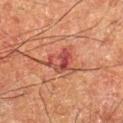This lesion was catalogued during total-body skin photography and was not selected for biopsy. The tile uses cross-polarized illumination. Approximately 4 mm at its widest. A close-up tile cropped from a whole-body skin photograph, about 15 mm across. A male patient, aged around 60. The lesion is located on the left forearm.